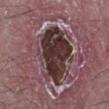biopsy status: total-body-photography surveillance lesion; no biopsy
acquisition: ~15 mm tile from a whole-body skin photo
subject: male, aged 48 to 52
automated metrics: a lesion area of about 25 mm², an eccentricity of roughly 0.7, and a shape-asymmetry score of about 0.25 (0 = symmetric); a mean CIELAB color near L≈32 a*≈18 b*≈16 and roughly 16 lightness units darker than nearby skin
anatomic site: the left lower leg
illumination: white-light illumination